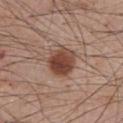A male subject about 60 years old.
A 15 mm close-up tile from a total-body photography series done for melanoma screening.
Approximately 3.5 mm at its widest.
Captured under white-light illumination.
Located on the abdomen.